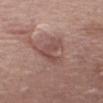  biopsy_status: not biopsied; imaged during a skin examination
  lesion_size:
    long_diameter_mm_approx: 4.0
  patient:
    sex: female
    age_approx: 50
  automated_metrics:
    eccentricity: 0.75
    cielab_L: 49
    cielab_a: 20
    cielab_b: 23
    vs_skin_darker_L: 8.0
    vs_skin_contrast_norm: 6.0
    peripheral_color_asymmetry: 1.0
    nevus_likeness_0_100: 5
    lesion_detection_confidence_0_100: 95
  site: abdomen
  image:
    source: total-body photography crop
    field_of_view_mm: 15
  lighting: white-light